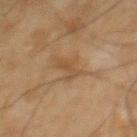Notes:
– follow-up · no biopsy performed (imaged during a skin exam)
– patient · male, roughly 65 years of age
– image source · ~15 mm tile from a whole-body skin photo
– lesion diameter · about 4 mm
– lighting · cross-polarized illumination
– anatomic site · the chest